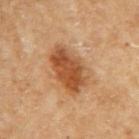The lesion was photographed on a routine skin check and not biopsied; there is no pathology result.
The patient is a female aged approximately 60.
On the right upper arm.
A roughly 15 mm field-of-view crop from a total-body skin photograph.